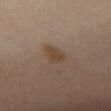Impression: Part of a total-body skin-imaging series; this lesion was reviewed on a skin check and was not flagged for biopsy. Context: The patient is a female roughly 35 years of age. The tile uses cross-polarized illumination. A region of skin cropped from a whole-body photographic capture, roughly 15 mm wide. The lesion-visualizer software estimated a footprint of about 5 mm², an eccentricity of roughly 0.85, and two-axis asymmetry of about 0.25. The software also gave a lesion–skin lightness drop of about 7. The software also gave a nevus-likeness score of about 30/100 and a detector confidence of about 100 out of 100 that the crop contains a lesion. Located on the abdomen. The lesion's longest dimension is about 3.5 mm.